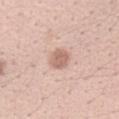| field | value |
|---|---|
| notes | catalogued during a skin exam; not biopsied |
| tile lighting | white-light |
| TBP lesion metrics | a footprint of about 5 mm², a shape eccentricity near 0.55, and two-axis asymmetry of about 0.15; an average lesion color of about L≈63 a*≈20 b*≈27 (CIELAB) and about 11 CIELAB-L* units darker than the surrounding skin |
| image | total-body-photography crop, ~15 mm field of view |
| body site | the arm |
| subject | female, aged around 40 |